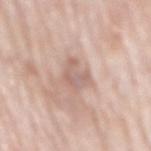workup = no biopsy performed (imaged during a skin exam); anatomic site = the mid back; subject = male, approximately 80 years of age; lesion size = about 3.5 mm; illumination = white-light; image = ~15 mm crop, total-body skin-cancer survey.